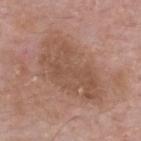Case summary:
* biopsy status: no biopsy performed (imaged during a skin exam)
* subject: male, in their mid- to late 60s
* anatomic site: the upper back
* illumination: white-light illumination
* imaging modality: 15 mm crop, total-body photography
* lesion diameter: about 9 mm